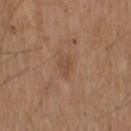workup: imaged on a skin check; not biopsied
tile lighting: white-light
size: about 2.5 mm
site: the left upper arm
acquisition: total-body-photography crop, ~15 mm field of view
patient: male, in their mid- to late 70s